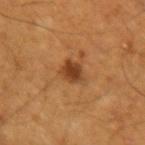{
  "biopsy_status": "not biopsied; imaged during a skin examination",
  "image": {
    "source": "total-body photography crop",
    "field_of_view_mm": 15
  },
  "lighting": "cross-polarized",
  "patient": {
    "sex": "male",
    "age_approx": 55
  },
  "site": "right upper arm"
}